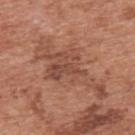  biopsy_status: not biopsied; imaged during a skin examination
  lighting: white-light
  lesion_size:
    long_diameter_mm_approx: 9.0
  patient:
    sex: male
    age_approx: 70
  site: upper back
  image:
    source: total-body photography crop
    field_of_view_mm: 15